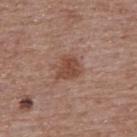Image and clinical context:
An algorithmic analysis of the crop reported roughly 10 lightness units darker than nearby skin and a normalized border contrast of about 8. The software also gave border irregularity of about 3 on a 0–10 scale, a color-variation rating of about 2/10, and a peripheral color-asymmetry measure near 1. The tile uses white-light illumination. A region of skin cropped from a whole-body photographic capture, roughly 15 mm wide. The lesion is on the back. The subject is a female approximately 65 years of age.